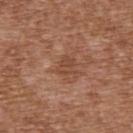- workup · total-body-photography surveillance lesion; no biopsy
- tile lighting · white-light illumination
- image · 15 mm crop, total-body photography
- patient · male, aged approximately 75
- body site · the upper back
- lesion diameter · ~2.5 mm (longest diameter)
- automated metrics · a mean CIELAB color near L≈46 a*≈22 b*≈30, roughly 7 lightness units darker than nearby skin, and a normalized lesion–skin contrast near 5.5; a border-irregularity index near 3.5/10 and a color-variation rating of about 2/10; a classifier nevus-likeness of about 0/100 and a lesion-detection confidence of about 100/100